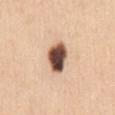Recorded during total-body skin imaging; not selected for excision or biopsy. On the chest. Longest diameter approximately 4 mm. A female subject, aged 28 to 32. A 15 mm close-up tile from a total-body photography series done for melanoma screening.